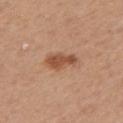workup: catalogued during a skin exam; not biopsied.